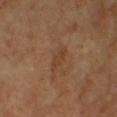biopsy_status: not biopsied; imaged during a skin examination
patient:
  sex: female
  age_approx: 55
image:
  source: total-body photography crop
  field_of_view_mm: 15
lighting: cross-polarized
site: right thigh